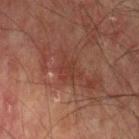Q: Was this lesion biopsied?
A: total-body-photography surveillance lesion; no biopsy
Q: Lesion size?
A: ≈3 mm
Q: Where on the body is the lesion?
A: the left upper arm
Q: What did automated image analysis measure?
A: a lesion color around L≈32 a*≈21 b*≈24 in CIELAB and a lesion-to-skin contrast of about 5 (normalized; higher = more distinct)
Q: Patient demographics?
A: male, aged around 75
Q: Illumination type?
A: cross-polarized
Q: What kind of image is this?
A: 15 mm crop, total-body photography